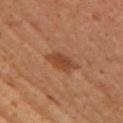This lesion was catalogued during total-body skin photography and was not selected for biopsy.
Measured at roughly 3.5 mm in maximum diameter.
Captured under cross-polarized illumination.
From the right upper arm.
The patient is a female about 40 years old.
Cropped from a total-body skin-imaging series; the visible field is about 15 mm.